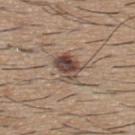biopsy_status: not biopsied; imaged during a skin examination
patient:
  sex: male
  age_approx: 60
image:
  source: total-body photography crop
  field_of_view_mm: 15
lesion_size:
  long_diameter_mm_approx: 4.0
lighting: white-light
automated_metrics:
  eccentricity: 0.7
  shape_asymmetry: 0.3
  color_variation_0_10: 10.0
  peripheral_color_asymmetry: 3.5
  nevus_likeness_0_100: 95
  lesion_detection_confidence_0_100: 100
site: upper back